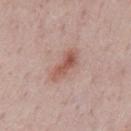Clinical impression:
The lesion was photographed on a routine skin check and not biopsied; there is no pathology result.